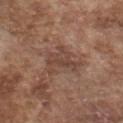A male subject, approximately 75 years of age. The total-body-photography lesion software estimated a footprint of about 9 mm², an outline eccentricity of about 0.7 (0 = round, 1 = elongated), and a symmetry-axis asymmetry near 0.5. This is a white-light tile. The lesion is located on the chest. Approximately 4.5 mm at its widest. Cropped from a whole-body photographic skin survey; the tile spans about 15 mm.